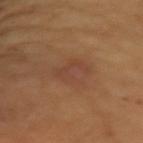The lesion is located on the arm. Measured at roughly 3.5 mm in maximum diameter. Automated image analysis of the tile measured a shape eccentricity near 0.55. And it measured a nevus-likeness score of about 10/100. Imaged with cross-polarized lighting. Cropped from a whole-body photographic skin survey; the tile spans about 15 mm. A female subject, about 55 years old.